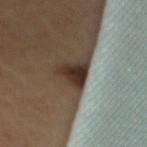<tbp_lesion>
  <biopsy_status>not biopsied; imaged during a skin examination</biopsy_status>
  <lighting>cross-polarized</lighting>
  <site>upper back</site>
  <image>
    <source>total-body photography crop</source>
    <field_of_view_mm>15</field_of_view_mm>
  </image>
  <lesion_size>
    <long_diameter_mm_approx>4.0</long_diameter_mm_approx>
  </lesion_size>
  <automated_metrics>
    <border_irregularity_0_10>4.0</border_irregularity_0_10>
    <color_variation_0_10>5.0</color_variation_0_10>
    <peripheral_color_asymmetry>1.5</peripheral_color_asymmetry>
    <nevus_likeness_0_100>5</nevus_likeness_0_100>
    <lesion_detection_confidence_0_100>100</lesion_detection_confidence_0_100>
  </automated_metrics>
  <patient>
    <sex>female</sex>
    <age_approx>60</age_approx>
  </patient>
</tbp_lesion>